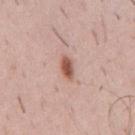biopsy status — total-body-photography surveillance lesion; no biopsy | subject — male, in their 50s | acquisition — ~15 mm crop, total-body skin-cancer survey | lighting — white-light | anatomic site — the chest.